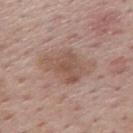{"biopsy_status": "not biopsied; imaged during a skin examination", "site": "mid back", "image": {"source": "total-body photography crop", "field_of_view_mm": 15}, "patient": {"sex": "male", "age_approx": 75}}